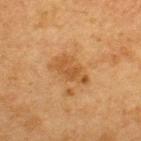No biopsy was performed on this lesion — it was imaged during a full skin examination and was not determined to be concerning.
Cropped from a whole-body photographic skin survey; the tile spans about 15 mm.
The lesion is located on the back.
The lesion's longest dimension is about 4.5 mm.
A male patient aged 73 to 77.
The total-body-photography lesion software estimated a footprint of about 7.5 mm², an eccentricity of roughly 0.9, and a symmetry-axis asymmetry near 0.35. The analysis additionally found a mean CIELAB color near L≈42 a*≈19 b*≈35, a lesion–skin lightness drop of about 7, and a normalized lesion–skin contrast near 6.5. It also reported a border-irregularity rating of about 4.5/10, a within-lesion color-variation index near 2/10, and peripheral color asymmetry of about 0.5. The analysis additionally found a nevus-likeness score of about 10/100.